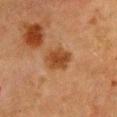Assessment: The lesion was photographed on a routine skin check and not biopsied; there is no pathology result. Context: A female subject, aged 48 to 52. Cropped from a whole-body photographic skin survey; the tile spans about 15 mm. The lesion is located on the chest.